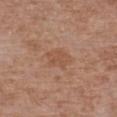Clinical impression: Captured during whole-body skin photography for melanoma surveillance; the lesion was not biopsied. Background: The lesion is located on the chest. A lesion tile, about 15 mm wide, cut from a 3D total-body photograph. Longest diameter approximately 3.5 mm. A female patient aged 73–77. Imaged with white-light lighting.